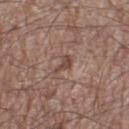Clinical impression:
The lesion was photographed on a routine skin check and not biopsied; there is no pathology result.
Clinical summary:
A male patient approximately 60 years of age. Approximately 3 mm at its widest. On the left thigh. A 15 mm close-up tile from a total-body photography series done for melanoma screening. This is a white-light tile.